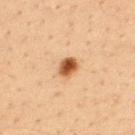Assessment: This lesion was catalogued during total-body skin photography and was not selected for biopsy. Clinical summary: Imaged with cross-polarized lighting. Located on the upper back. A male subject, aged 33–37. A lesion tile, about 15 mm wide, cut from a 3D total-body photograph. Approximately 2.5 mm at its widest.